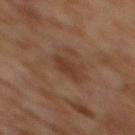The lesion was photographed on a routine skin check and not biopsied; there is no pathology result.
A 15 mm close-up extracted from a 3D total-body photography capture.
The lesion is on the upper back.
A female subject, aged approximately 60.